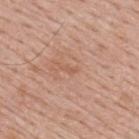Imaged during a routine full-body skin examination; the lesion was not biopsied and no histopathology is available. The lesion is on the upper back. The subject is a male aged 38–42. Measured at roughly 2 mm in maximum diameter. The tile uses white-light illumination. A roughly 15 mm field-of-view crop from a total-body skin photograph. The lesion-visualizer software estimated an area of roughly 1 mm², an outline eccentricity of about 0.95 (0 = round, 1 = elongated), and two-axis asymmetry of about 0.45. The software also gave a lesion color around L≈57 a*≈22 b*≈31 in CIELAB, a lesion–skin lightness drop of about 6, and a normalized border contrast of about 4.5. The software also gave a within-lesion color-variation index near 0/10 and a peripheral color-asymmetry measure near 0.